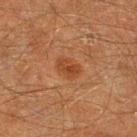{
  "image": {
    "source": "total-body photography crop",
    "field_of_view_mm": 15
  },
  "automated_metrics": {
    "area_mm2_approx": 5.0,
    "eccentricity": 0.75,
    "shape_asymmetry": 0.2,
    "cielab_L": 34,
    "cielab_a": 21,
    "cielab_b": 30,
    "vs_skin_darker_L": 7.0,
    "vs_skin_contrast_norm": 6.5,
    "border_irregularity_0_10": 2.0,
    "peripheral_color_asymmetry": 1.0
  },
  "lighting": "cross-polarized",
  "patient": {
    "sex": "male",
    "age_approx": 60
  },
  "site": "right lower leg"
}